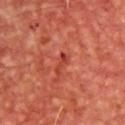{"biopsy_status": "not biopsied; imaged during a skin examination", "site": "chest", "patient": {"sex": "male", "age_approx": 65}, "image": {"source": "total-body photography crop", "field_of_view_mm": 15}}